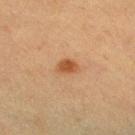notes: catalogued during a skin exam; not biopsied | imaging modality: total-body-photography crop, ~15 mm field of view | tile lighting: cross-polarized | subject: female, aged approximately 40 | lesion diameter: ≈2.5 mm | site: the right lower leg.